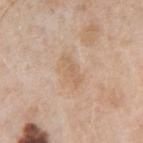Recorded during total-body skin imaging; not selected for excision or biopsy.
The total-body-photography lesion software estimated a lesion color around L≈63 a*≈17 b*≈32 in CIELAB. It also reported a color-variation rating of about 1.5/10 and peripheral color asymmetry of about 0.5. The analysis additionally found a classifier nevus-likeness of about 0/100 and a lesion-detection confidence of about 100/100.
Measured at roughly 4 mm in maximum diameter.
A male subject, about 55 years old.
The lesion is located on the left upper arm.
A roughly 15 mm field-of-view crop from a total-body skin photograph.
This is a white-light tile.